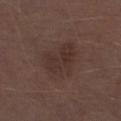Notes:
- biopsy status: total-body-photography surveillance lesion; no biopsy
- size: ≈5 mm
- lighting: white-light
- subject: male, aged 68 to 72
- anatomic site: the left lower leg
- image source: 15 mm crop, total-body photography
- TBP lesion metrics: a nevus-likeness score of about 5/100 and a detector confidence of about 100 out of 100 that the crop contains a lesion